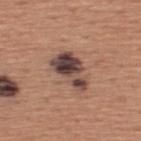On the upper back.
A region of skin cropped from a whole-body photographic capture, roughly 15 mm wide.
The subject is a male in their mid-40s.